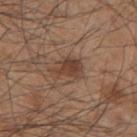follow-up = total-body-photography surveillance lesion; no biopsy
acquisition = total-body-photography crop, ~15 mm field of view
body site = the right upper arm
diameter = ≈4 mm
tile lighting = white-light illumination
patient = male, roughly 45 years of age